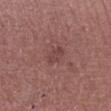Case summary:
* biopsy status — imaged on a skin check; not biopsied
* subject — male, aged approximately 65
* location — the leg
* acquisition — 15 mm crop, total-body photography